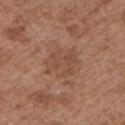| feature | finding |
|---|---|
| follow-up | total-body-photography surveillance lesion; no biopsy |
| image | 15 mm crop, total-body photography |
| location | the left upper arm |
| patient | male, in their mid- to late 70s |
| lesion diameter | ~5 mm (longest diameter) |
| lighting | white-light |